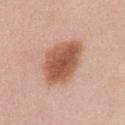notes = catalogued during a skin exam; not biopsied
image = total-body-photography crop, ~15 mm field of view
subject = female, approximately 50 years of age
location = the front of the torso